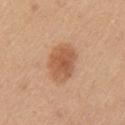A female patient aged 43 to 47.
The lesion is located on the left upper arm.
Measured at roughly 4.5 mm in maximum diameter.
A 15 mm crop from a total-body photograph taken for skin-cancer surveillance.